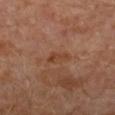biopsy status = catalogued during a skin exam; not biopsied | lesion diameter = about 3 mm | automated metrics = a lesion area of about 3 mm²; a mean CIELAB color near L≈40 a*≈22 b*≈31; a nevus-likeness score of about 0/100 | patient = male, aged around 30 | image source = ~15 mm crop, total-body skin-cancer survey | illumination = cross-polarized illumination | anatomic site = the left leg.